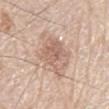Captured during whole-body skin photography for melanoma surveillance; the lesion was not biopsied.
A roughly 15 mm field-of-view crop from a total-body skin photograph.
This is a white-light tile.
The subject is a male about 80 years old.
The lesion-visualizer software estimated a lesion area of about 14 mm², an eccentricity of roughly 0.65, and a symmetry-axis asymmetry near 0.2. The software also gave border irregularity of about 2.5 on a 0–10 scale, a color-variation rating of about 5/10, and radial color variation of about 1.5. It also reported a classifier nevus-likeness of about 20/100.
Located on the mid back.